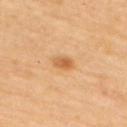automated lesion analysis: a mean CIELAB color near L≈64 a*≈23 b*≈44, roughly 10 lightness units darker than nearby skin, and a lesion-to-skin contrast of about 7 (normalized; higher = more distinct); a classifier nevus-likeness of about 90/100 and a lesion-detection confidence of about 100/100 | size: about 2.5 mm | image source: total-body-photography crop, ~15 mm field of view | patient: female, in their 60s | location: the upper back | tile lighting: cross-polarized.